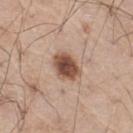notes — catalogued during a skin exam; not biopsied
diameter — ~4 mm (longest diameter)
TBP lesion metrics — an outline eccentricity of about 0.7 (0 = round, 1 = elongated) and two-axis asymmetry of about 0.15; a border-irregularity index near 1.5/10 and a within-lesion color-variation index near 4.5/10; a classifier nevus-likeness of about 100/100 and a lesion-detection confidence of about 100/100
patient — male, aged 68–72
site — the right thigh
image source — ~15 mm crop, total-body skin-cancer survey